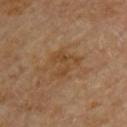biopsy_status: not biopsied; imaged during a skin examination
image:
  source: total-body photography crop
  field_of_view_mm: 15
site: upper back
patient:
  sex: male
  age_approx: 85+
automated_metrics:
  cielab_L: 43
  cielab_a: 18
  cielab_b: 33
  vs_skin_darker_L: 6.0
  vs_skin_contrast_norm: 6.0
  nevus_likeness_0_100: 0
  lesion_detection_confidence_0_100: 100
lighting: cross-polarized
lesion_size:
  long_diameter_mm_approx: 4.5